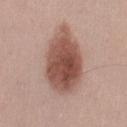Notes:
- follow-up — catalogued during a skin exam; not biopsied
- body site — the left thigh
- image source — total-body-photography crop, ~15 mm field of view
- patient — female, about 40 years old
- size — about 8 mm
- tile lighting — white-light illumination